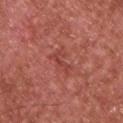The lesion was tiled from a total-body skin photograph and was not biopsied. The lesion is on the upper back. The tile uses white-light illumination. An algorithmic analysis of the crop reported a lesion area of about 4 mm², a shape eccentricity near 0.9, and a shape-asymmetry score of about 0.55 (0 = symmetric). It also reported border irregularity of about 7 on a 0–10 scale, internal color variation of about 2 on a 0–10 scale, and a peripheral color-asymmetry measure near 1. A 15 mm close-up extracted from a 3D total-body photography capture. The lesion's longest dimension is about 3.5 mm. A male patient about 65 years old.